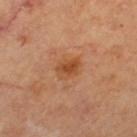Impression: No biopsy was performed on this lesion — it was imaged during a full skin examination and was not determined to be concerning. Acquisition and patient details: Measured at roughly 3 mm in maximum diameter. From the leg. An algorithmic analysis of the crop reported a lesion area of about 5 mm². It also reported a mean CIELAB color near L≈50 a*≈26 b*≈39 and a lesion-to-skin contrast of about 7.5 (normalized; higher = more distinct). And it measured an automated nevus-likeness rating near 65 out of 100 and a detector confidence of about 100 out of 100 that the crop contains a lesion. A female subject, approximately 60 years of age. A 15 mm crop from a total-body photograph taken for skin-cancer surveillance.